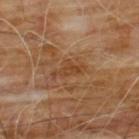workup: catalogued during a skin exam; not biopsied
lighting: cross-polarized illumination
patient: male, aged approximately 60
image source: ~15 mm tile from a whole-body skin photo
location: the chest